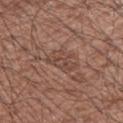<record>
  <biopsy_status>not biopsied; imaged during a skin examination</biopsy_status>
  <lighting>white-light</lighting>
  <patient>
    <sex>male</sex>
    <age_approx>65</age_approx>
  </patient>
  <image>
    <source>total-body photography crop</source>
    <field_of_view_mm>15</field_of_view_mm>
  </image>
  <site>right upper arm</site>
  <lesion_size>
    <long_diameter_mm_approx>3.5</long_diameter_mm_approx>
  </lesion_size>
</record>